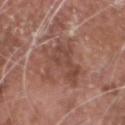{
  "biopsy_status": "not biopsied; imaged during a skin examination",
  "patient": {
    "sex": "male",
    "age_approx": 75
  },
  "lighting": "white-light",
  "image": {
    "source": "total-body photography crop",
    "field_of_view_mm": 15
  },
  "lesion_size": {
    "long_diameter_mm_approx": 6.0
  },
  "site": "head or neck"
}